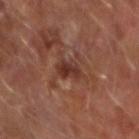This lesion was catalogued during total-body skin photography and was not selected for biopsy.
Cropped from a whole-body photographic skin survey; the tile spans about 15 mm.
A male subject, aged 63 to 67.
The lesion is located on the right forearm.